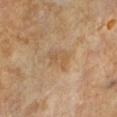workup = no biopsy performed (imaged during a skin exam); tile lighting = cross-polarized illumination; subject = male, in their mid-60s; image source = ~15 mm tile from a whole-body skin photo; location = the left lower leg.